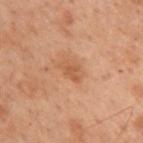| field | value |
|---|---|
| workup | catalogued during a skin exam; not biopsied |
| image | total-body-photography crop, ~15 mm field of view |
| patient | female, aged 53 to 57 |
| body site | the upper back |
| automated metrics | a lesion color around L≈46 a*≈21 b*≈31 in CIELAB, a lesion–skin lightness drop of about 7, and a normalized lesion–skin contrast near 5.5 |
| lighting | cross-polarized |
| diameter | about 2.5 mm |